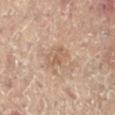Clinical impression: The lesion was photographed on a routine skin check and not biopsied; there is no pathology result. Image and clinical context: From the left leg. The lesion's longest dimension is about 3 mm. A lesion tile, about 15 mm wide, cut from a 3D total-body photograph. The patient is a female aged 78–82. This is a cross-polarized tile. An algorithmic analysis of the crop reported a footprint of about 3 mm² and an eccentricity of roughly 0.85. The software also gave a lesion color around L≈52 a*≈16 b*≈27 in CIELAB and about 7 CIELAB-L* units darker than the surrounding skin. It also reported a border-irregularity index near 5.5/10, a within-lesion color-variation index near 0/10, and peripheral color asymmetry of about 0. And it measured a lesion-detection confidence of about 100/100.